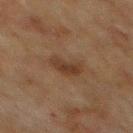Recorded during total-body skin imaging; not selected for excision or biopsy.
This is a cross-polarized tile.
Longest diameter approximately 3.5 mm.
A roughly 15 mm field-of-view crop from a total-body skin photograph.
Automated tile analysis of the lesion measured a lesion color around L≈30 a*≈15 b*≈25 in CIELAB and a lesion–skin lightness drop of about 7. It also reported a border-irregularity rating of about 3.5/10, internal color variation of about 3 on a 0–10 scale, and peripheral color asymmetry of about 1. And it measured an automated nevus-likeness rating near 30 out of 100 and lesion-presence confidence of about 100/100.
A male subject, aged 68 to 72.
The lesion is located on the mid back.